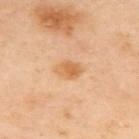follow-up: no biopsy performed (imaged during a skin exam) | lesion diameter: about 3 mm | site: the upper back | lighting: cross-polarized illumination | patient: female, about 45 years old | automated metrics: an average lesion color of about L≈65 a*≈23 b*≈43 (CIELAB), roughly 9 lightness units darker than nearby skin, and a lesion-to-skin contrast of about 7 (normalized; higher = more distinct); a border-irregularity rating of about 2/10, a color-variation rating of about 3/10, and radial color variation of about 1; an automated nevus-likeness rating near 30 out of 100 and a detector confidence of about 100 out of 100 that the crop contains a lesion | acquisition: ~15 mm crop, total-body skin-cancer survey.